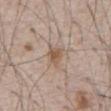| key | value |
|---|---|
| workup | total-body-photography surveillance lesion; no biopsy |
| image | ~15 mm crop, total-body skin-cancer survey |
| lesion diameter | ≈2.5 mm |
| site | the chest |
| tile lighting | white-light illumination |
| subject | male, aged approximately 65 |